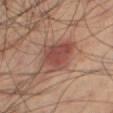The lesion was photographed on a routine skin check and not biopsied; there is no pathology result. From the right thigh. Approximately 5.5 mm at its widest. Imaged with cross-polarized lighting. The total-body-photography lesion software estimated internal color variation of about 6 on a 0–10 scale and a peripheral color-asymmetry measure near 2. The software also gave a classifier nevus-likeness of about 85/100 and lesion-presence confidence of about 100/100. The subject is a male about 60 years old. A 15 mm close-up tile from a total-body photography series done for melanoma screening.